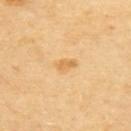Clinical summary:
A female patient aged around 70. From the upper back. Cropped from a whole-body photographic skin survey; the tile spans about 15 mm. The total-body-photography lesion software estimated a footprint of about 3.5 mm² and a shape eccentricity near 0.85. And it measured a lesion-detection confidence of about 100/100. Measured at roughly 2.5 mm in maximum diameter. The tile uses cross-polarized illumination.